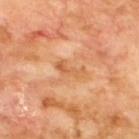{
  "biopsy_status": "not biopsied; imaged during a skin examination",
  "image": {
    "source": "total-body photography crop",
    "field_of_view_mm": 15
  },
  "lighting": "cross-polarized",
  "patient": {
    "sex": "male",
    "age_approx": 70
  },
  "site": "upper back"
}